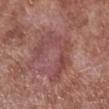notes — total-body-photography surveillance lesion; no biopsy | anatomic site — the leg | image source — ~15 mm crop, total-body skin-cancer survey | illumination — white-light illumination | subject — male, approximately 55 years of age | size — ≈6.5 mm.